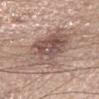<case>
  <biopsy_status>not biopsied; imaged during a skin examination</biopsy_status>
  <patient>
    <sex>female</sex>
    <age_approx>65</age_approx>
  </patient>
  <lighting>white-light</lighting>
  <lesion_size>
    <long_diameter_mm_approx>8.5</long_diameter_mm_approx>
  </lesion_size>
  <image>
    <source>total-body photography crop</source>
    <field_of_view_mm>15</field_of_view_mm>
  </image>
  <site>head or neck</site>
</case>